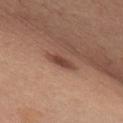biopsy status = imaged on a skin check; not biopsied
subject = female, aged 48–52
image = ~15 mm crop, total-body skin-cancer survey
site = the front of the torso